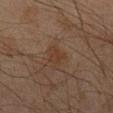Automated image analysis of the tile measured a within-lesion color-variation index near 1.5/10 and a peripheral color-asymmetry measure near 0.5. The analysis additionally found a classifier nevus-likeness of about 0/100 and a detector confidence of about 100 out of 100 that the crop contains a lesion.
Imaged with cross-polarized lighting.
Approximately 3 mm at its widest.
The lesion is on the back.
A male subject about 45 years old.
A close-up tile cropped from a whole-body skin photograph, about 15 mm across.